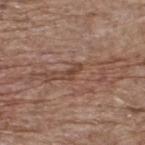Part of a total-body skin-imaging series; this lesion was reviewed on a skin check and was not flagged for biopsy. Cropped from a total-body skin-imaging series; the visible field is about 15 mm. Automated image analysis of the tile measured border irregularity of about 3 on a 0–10 scale and radial color variation of about 0. The software also gave a nevus-likeness score of about 0/100 and a detector confidence of about 55 out of 100 that the crop contains a lesion. Located on the upper back. The patient is a male aged 68–72.